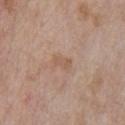| key | value |
|---|---|
| notes | total-body-photography surveillance lesion; no biopsy |